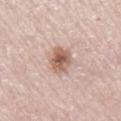Recorded during total-body skin imaging; not selected for excision or biopsy.
A male patient, aged approximately 80.
Imaged with white-light lighting.
Approximately 3.5 mm at its widest.
A 15 mm close-up extracted from a 3D total-body photography capture.
The lesion is on the leg.